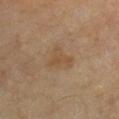biopsy status: imaged on a skin check; not biopsied
subject: male, roughly 70 years of age
anatomic site: the chest
tile lighting: cross-polarized
lesion diameter: ≈3 mm
automated lesion analysis: a lesion area of about 6 mm² and an outline eccentricity of about 0.55 (0 = round, 1 = elongated); an average lesion color of about L≈46 a*≈15 b*≈31 (CIELAB) and a lesion-to-skin contrast of about 5.5 (normalized; higher = more distinct); border irregularity of about 5.5 on a 0–10 scale and a within-lesion color-variation index near 2/10
acquisition: 15 mm crop, total-body photography